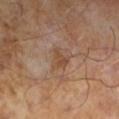Captured during whole-body skin photography for melanoma surveillance; the lesion was not biopsied. A close-up tile cropped from a whole-body skin photograph, about 15 mm across. The patient is a male aged approximately 65.